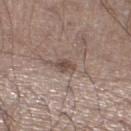notes = catalogued during a skin exam; not biopsied.